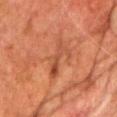This image is a 15 mm lesion crop taken from a total-body photograph.
A male patient approximately 75 years of age.
The lesion is on the front of the torso.
About 5 mm across.
The tile uses cross-polarized illumination.